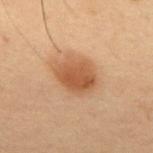Q: Was a biopsy performed?
A: no biopsy performed (imaged during a skin exam)
Q: Who is the patient?
A: male, aged approximately 55
Q: Automated lesion metrics?
A: an area of roughly 12 mm² and an outline eccentricity of about 0.55 (0 = round, 1 = elongated); a lesion–skin lightness drop of about 10 and a normalized lesion–skin contrast near 8; border irregularity of about 2 on a 0–10 scale, a within-lesion color-variation index near 3.5/10, and peripheral color asymmetry of about 1
Q: What is the imaging modality?
A: 15 mm crop, total-body photography
Q: What lighting was used for the tile?
A: cross-polarized illumination
Q: Lesion location?
A: the back